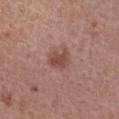Assessment:
Captured during whole-body skin photography for melanoma surveillance; the lesion was not biopsied.
Acquisition and patient details:
The tile uses white-light illumination. Cropped from a total-body skin-imaging series; the visible field is about 15 mm. On the left forearm. The subject is a female aged approximately 40. About 3 mm across. The lesion-visualizer software estimated an area of roughly 5.5 mm², a shape eccentricity near 0.75, and a symmetry-axis asymmetry near 0.25. And it measured an average lesion color of about L≈48 a*≈22 b*≈26 (CIELAB), about 9 CIELAB-L* units darker than the surrounding skin, and a lesion-to-skin contrast of about 7 (normalized; higher = more distinct). The software also gave border irregularity of about 2.5 on a 0–10 scale, internal color variation of about 2 on a 0–10 scale, and radial color variation of about 0.5.